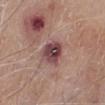Impression:
Imaged during a routine full-body skin examination; the lesion was not biopsied and no histopathology is available.
Context:
This is a white-light tile. Cropped from a total-body skin-imaging series; the visible field is about 15 mm. Located on the left lower leg. The recorded lesion diameter is about 4 mm. A male patient aged 63 to 67.